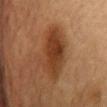Notes:
* notes · total-body-photography surveillance lesion; no biopsy
* image-analysis metrics · border irregularity of about 3 on a 0–10 scale, a color-variation rating of about 4.5/10, and radial color variation of about 1.5
* size · ~7 mm (longest diameter)
* image · total-body-photography crop, ~15 mm field of view
* location · the front of the torso
* patient · male, aged approximately 85 or older
* tile lighting · cross-polarized